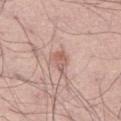<case>
  <biopsy_status>not biopsied; imaged during a skin examination</biopsy_status>
  <site>right thigh</site>
  <patient>
    <sex>male</sex>
    <age_approx>50</age_approx>
  </patient>
  <lesion_size>
    <long_diameter_mm_approx>2.5</long_diameter_mm_approx>
  </lesion_size>
  <image>
    <source>total-body photography crop</source>
    <field_of_view_mm>15</field_of_view_mm>
  </image>
  <automated_metrics>
    <border_irregularity_0_10>3.5</border_irregularity_0_10>
    <color_variation_0_10>3.5</color_variation_0_10>
    <nevus_likeness_0_100>15</nevus_likeness_0_100>
    <lesion_detection_confidence_0_100>100</lesion_detection_confidence_0_100>
  </automated_metrics>
</case>